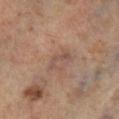Impression:
This lesion was catalogued during total-body skin photography and was not selected for biopsy.
Background:
Automated image analysis of the tile measured an average lesion color of about L≈51 a*≈18 b*≈25 (CIELAB), roughly 7 lightness units darker than nearby skin, and a normalized border contrast of about 5.5. The software also gave a border-irregularity index near 6.5/10 and internal color variation of about 0 on a 0–10 scale. Imaged with cross-polarized lighting. Cropped from a whole-body photographic skin survey; the tile spans about 15 mm. From the right lower leg. Approximately 3 mm at its widest.